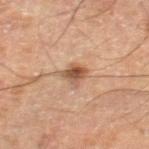No biopsy was performed on this lesion — it was imaged during a full skin examination and was not determined to be concerning. A 15 mm crop from a total-body photograph taken for skin-cancer surveillance. A male subject, aged approximately 60. On the right thigh. Longest diameter approximately 2.5 mm.